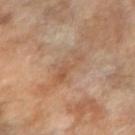follow-up: catalogued during a skin exam; not biopsied | lesion diameter: ≈4 mm | body site: the right forearm | image source: ~15 mm tile from a whole-body skin photo | subject: female, approximately 70 years of age | illumination: cross-polarized illumination.